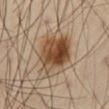Imaged during a routine full-body skin examination; the lesion was not biopsied and no histopathology is available. A male subject, aged 53–57. The lesion is on the abdomen. About 6 mm across. A 15 mm crop from a total-body photograph taken for skin-cancer surveillance.